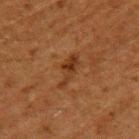Findings:
• lesion diameter: ≈4 mm
• image: ~15 mm crop, total-body skin-cancer survey
• TBP lesion metrics: a lesion area of about 4.5 mm², a shape eccentricity near 0.95, and a shape-asymmetry score of about 0.5 (0 = symmetric); internal color variation of about 2 on a 0–10 scale and radial color variation of about 0.5
• site: the upper back
• lighting: cross-polarized illumination
• patient: male, aged around 60